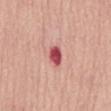Impression: No biopsy was performed on this lesion — it was imaged during a full skin examination and was not determined to be concerning.